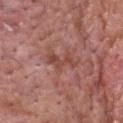biopsy status=imaged on a skin check; not biopsied | body site=the head or neck | subject=male, about 60 years old | image=~15 mm tile from a whole-body skin photo | lighting=white-light illumination.